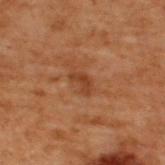This lesion was catalogued during total-body skin photography and was not selected for biopsy. The lesion is located on the upper back. A male subject, aged approximately 70. A lesion tile, about 15 mm wide, cut from a 3D total-body photograph.